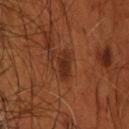tile lighting — cross-polarized
site — the head or neck
lesion size — ≈3.5 mm
automated metrics — a lesion area of about 5 mm², an outline eccentricity of about 0.85 (0 = round, 1 = elongated), and two-axis asymmetry of about 0.2; a border-irregularity rating of about 1.5/10; a classifier nevus-likeness of about 25/100
patient — male, in their mid- to late 50s
imaging modality — ~15 mm crop, total-body skin-cancer survey